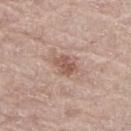Part of a total-body skin-imaging series; this lesion was reviewed on a skin check and was not flagged for biopsy. The lesion's longest dimension is about 3 mm. Captured under white-light illumination. The subject is a male aged around 80. A close-up tile cropped from a whole-body skin photograph, about 15 mm across. Automated image analysis of the tile measured a lesion area of about 5 mm², an eccentricity of roughly 0.75, and a symmetry-axis asymmetry near 0.25. It also reported an average lesion color of about L≈55 a*≈20 b*≈26 (CIELAB) and about 10 CIELAB-L* units darker than the surrounding skin. The analysis additionally found a border-irregularity index near 3/10, internal color variation of about 2.5 on a 0–10 scale, and a peripheral color-asymmetry measure near 1. The analysis additionally found a nevus-likeness score of about 65/100 and a lesion-detection confidence of about 100/100. From the leg.